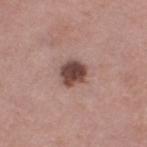| field | value |
|---|---|
| follow-up | imaged on a skin check; not biopsied |
| lesion size | ≈3 mm |
| TBP lesion metrics | an outline eccentricity of about 0.3 (0 = round, 1 = elongated) and a symmetry-axis asymmetry near 0.2; a lesion color around L≈45 a*≈20 b*≈22 in CIELAB, a lesion–skin lightness drop of about 17, and a lesion-to-skin contrast of about 12 (normalized; higher = more distinct); a border-irregularity index near 1.5/10, internal color variation of about 4.5 on a 0–10 scale, and peripheral color asymmetry of about 1.5; a lesion-detection confidence of about 100/100 |
| subject | female, aged 68 to 72 |
| lighting | white-light |
| image | 15 mm crop, total-body photography |
| body site | the right thigh |